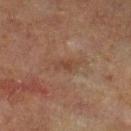Impression: Recorded during total-body skin imaging; not selected for excision or biopsy. Clinical summary: A male patient roughly 65 years of age. Longest diameter approximately 2.5 mm. The tile uses cross-polarized illumination. A roughly 15 mm field-of-view crop from a total-body skin photograph. Automated tile analysis of the lesion measured an area of roughly 2.5 mm² and an outline eccentricity of about 0.9 (0 = round, 1 = elongated). And it measured a lesion color around L≈33 a*≈16 b*≈24 in CIELAB, about 5 CIELAB-L* units darker than the surrounding skin, and a lesion-to-skin contrast of about 5.5 (normalized; higher = more distinct). And it measured a border-irregularity index near 3/10, a color-variation rating of about 0.5/10, and radial color variation of about 0. The software also gave a classifier nevus-likeness of about 0/100. Located on the left lower leg.